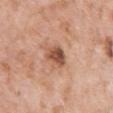Q: Is there a histopathology result?
A: imaged on a skin check; not biopsied
Q: What is the anatomic site?
A: the arm
Q: Patient demographics?
A: female, aged 68 to 72
Q: What is the imaging modality?
A: ~15 mm crop, total-body skin-cancer survey